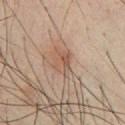{
  "biopsy_status": "not biopsied; imaged during a skin examination",
  "patient": {
    "sex": "male",
    "age_approx": 50
  },
  "lesion_size": {
    "long_diameter_mm_approx": 3.5
  },
  "site": "chest",
  "automated_metrics": {
    "cielab_L": 48,
    "cielab_a": 17,
    "cielab_b": 26,
    "vs_skin_darker_L": 7.0,
    "color_variation_0_10": 4.5,
    "peripheral_color_asymmetry": 1.5
  },
  "image": {
    "source": "total-body photography crop",
    "field_of_view_mm": 15
  }
}